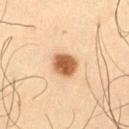This lesion was catalogued during total-body skin photography and was not selected for biopsy. Approximately 3 mm at its widest. The tile uses cross-polarized illumination. The subject is a male aged 63 to 67. A close-up tile cropped from a whole-body skin photograph, about 15 mm across. From the left thigh. The lesion-visualizer software estimated a lesion-to-skin contrast of about 11 (normalized; higher = more distinct).